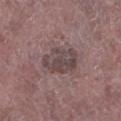biopsy_status: not biopsied; imaged during a skin examination
patient:
  sex: male
  age_approx: 75
image:
  source: total-body photography crop
  field_of_view_mm: 15
site: left lower leg
lesion_size:
  long_diameter_mm_approx: 4.5